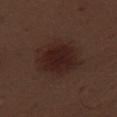follow-up = catalogued during a skin exam; not biopsied
size = ≈6.5 mm
patient = male, approximately 70 years of age
site = the right thigh
acquisition = ~15 mm tile from a whole-body skin photo
illumination = white-light illumination